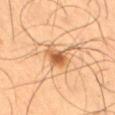biopsy_status: not biopsied; imaged during a skin examination
lighting: cross-polarized
site: leg
patient:
  sex: male
  age_approx: 55
image:
  source: total-body photography crop
  field_of_view_mm: 15